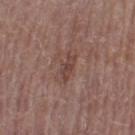Q: Was this lesion biopsied?
A: imaged on a skin check; not biopsied
Q: What did automated image analysis measure?
A: a mean CIELAB color near L≈43 a*≈19 b*≈22, about 8 CIELAB-L* units darker than the surrounding skin, and a lesion-to-skin contrast of about 6 (normalized; higher = more distinct); a border-irregularity index near 4.5/10, a color-variation rating of about 1/10, and a peripheral color-asymmetry measure near 0.5; a classifier nevus-likeness of about 0/100 and lesion-presence confidence of about 100/100
Q: What is the lesion's diameter?
A: ≈3 mm
Q: What lighting was used for the tile?
A: white-light
Q: What is the imaging modality?
A: total-body-photography crop, ~15 mm field of view
Q: Patient demographics?
A: female, aged 48–52
Q: Where on the body is the lesion?
A: the left thigh